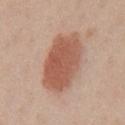notes: no biopsy performed (imaged during a skin exam)
illumination: white-light illumination
patient: male, roughly 60 years of age
lesion size: ~7.5 mm (longest diameter)
body site: the chest
image source: ~15 mm crop, total-body skin-cancer survey